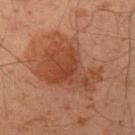| field | value |
|---|---|
| follow-up | no biopsy performed (imaged during a skin exam) |
| subject | male, roughly 50 years of age |
| image | 15 mm crop, total-body photography |
| body site | the left upper arm |